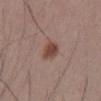Q: Who is the patient?
A: male, roughly 55 years of age
Q: Where on the body is the lesion?
A: the abdomen
Q: Illumination type?
A: white-light illumination
Q: How was this image acquired?
A: 15 mm crop, total-body photography
Q: What did automated image analysis measure?
A: roughly 11 lightness units darker than nearby skin and a normalized border contrast of about 9; a within-lesion color-variation index near 4/10 and radial color variation of about 1; an automated nevus-likeness rating near 100 out of 100 and a detector confidence of about 100 out of 100 that the crop contains a lesion